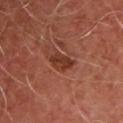Q: Lesion size?
A: ~3.5 mm (longest diameter)
Q: What kind of image is this?
A: 15 mm crop, total-body photography
Q: What is the anatomic site?
A: the chest
Q: How was the tile lit?
A: cross-polarized illumination
Q: Who is the patient?
A: male, aged around 50